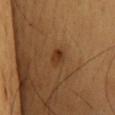Image and clinical context:
Measured at roughly 2.5 mm in maximum diameter. A 15 mm close-up tile from a total-body photography series done for melanoma screening. The patient is a male aged around 60. Captured under cross-polarized illumination. Located on the mid back.